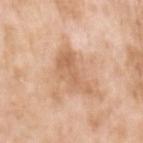notes: total-body-photography surveillance lesion; no biopsy | lighting: white-light | image: total-body-photography crop, ~15 mm field of view | patient: female, in their mid- to late 70s | location: the left upper arm | lesion diameter: about 5 mm | automated lesion analysis: an eccentricity of roughly 0.85 and a shape-asymmetry score of about 0.5 (0 = symmetric); an average lesion color of about L≈63 a*≈21 b*≈34 (CIELAB), about 9 CIELAB-L* units darker than the surrounding skin, and a normalized border contrast of about 6; a color-variation rating of about 3/10 and radial color variation of about 1.